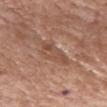notes = catalogued during a skin exam; not biopsied | automated lesion analysis = a border-irregularity index near 4/10, a within-lesion color-variation index near 4/10, and a peripheral color-asymmetry measure near 1.5; an automated nevus-likeness rating near 0 out of 100 and a detector confidence of about 60 out of 100 that the crop contains a lesion | site = the head or neck | image = total-body-photography crop, ~15 mm field of view | lesion diameter = about 5 mm | subject = female, aged approximately 70.